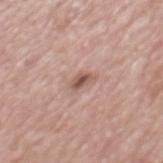• workup — catalogued during a skin exam; not biopsied
• imaging modality — ~15 mm crop, total-body skin-cancer survey
• patient — male, aged 68–72
• illumination — white-light
• lesion diameter — ≈2.5 mm
• location — the mid back